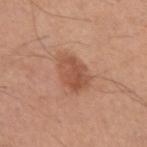Assessment: Captured during whole-body skin photography for melanoma surveillance; the lesion was not biopsied. Context: Imaged with white-light lighting. The subject is a male roughly 55 years of age. From the left upper arm. The lesion's longest dimension is about 4.5 mm. An algorithmic analysis of the crop reported a lesion area of about 11 mm², an outline eccentricity of about 0.6 (0 = round, 1 = elongated), and a shape-asymmetry score of about 0.2 (0 = symmetric). It also reported a lesion color around L≈53 a*≈23 b*≈32 in CIELAB, roughly 10 lightness units darker than nearby skin, and a lesion-to-skin contrast of about 7 (normalized; higher = more distinct). Cropped from a whole-body photographic skin survey; the tile spans about 15 mm.